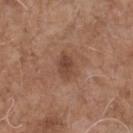Q: Was this lesion biopsied?
A: no biopsy performed (imaged during a skin exam)
Q: Patient demographics?
A: male, aged around 70
Q: What is the lesion's diameter?
A: about 3 mm
Q: What kind of image is this?
A: total-body-photography crop, ~15 mm field of view
Q: What did automated image analysis measure?
A: a lesion area of about 4.5 mm² and a symmetry-axis asymmetry near 0.2; a border-irregularity index near 2/10, a within-lesion color-variation index near 3/10, and a peripheral color-asymmetry measure near 1; a classifier nevus-likeness of about 5/100 and a lesion-detection confidence of about 100/100
Q: How was the tile lit?
A: white-light illumination
Q: Where on the body is the lesion?
A: the chest